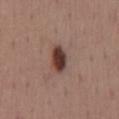Q: Is there a histopathology result?
A: catalogued during a skin exam; not biopsied
Q: Where on the body is the lesion?
A: the mid back
Q: Illumination type?
A: white-light illumination
Q: What is the imaging modality?
A: ~15 mm tile from a whole-body skin photo
Q: Automated lesion metrics?
A: a lesion color around L≈39 a*≈19 b*≈23 in CIELAB, a lesion–skin lightness drop of about 16, and a lesion-to-skin contrast of about 12.5 (normalized; higher = more distinct); border irregularity of about 1.5 on a 0–10 scale and internal color variation of about 5 on a 0–10 scale; an automated nevus-likeness rating near 100 out of 100
Q: Patient demographics?
A: female, aged 48 to 52
Q: Lesion size?
A: ≈3.5 mm